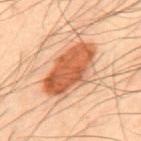Clinical impression:
No biopsy was performed on this lesion — it was imaged during a full skin examination and was not determined to be concerning.
Context:
Located on the upper back. A male patient about 50 years old. Imaged with cross-polarized lighting. A roughly 15 mm field-of-view crop from a total-body skin photograph.